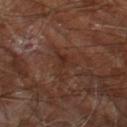Q: Was this lesion biopsied?
A: total-body-photography surveillance lesion; no biopsy
Q: How was this image acquired?
A: ~15 mm crop, total-body skin-cancer survey
Q: Lesion location?
A: the right leg
Q: Who is the patient?
A: male, aged around 60
Q: How was the tile lit?
A: cross-polarized illumination